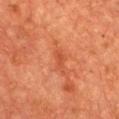The lesion's longest dimension is about 2.5 mm.
Automated tile analysis of the lesion measured an average lesion color of about L≈44 a*≈30 b*≈36 (CIELAB), roughly 6 lightness units darker than nearby skin, and a normalized lesion–skin contrast near 5. It also reported lesion-presence confidence of about 100/100.
A lesion tile, about 15 mm wide, cut from a 3D total-body photograph.
The lesion is on the front of the torso.
A female patient in their 70s.